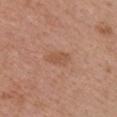<lesion>
<biopsy_status>not biopsied; imaged during a skin examination</biopsy_status>
<image>
  <source>total-body photography crop</source>
  <field_of_view_mm>15</field_of_view_mm>
</image>
<lighting>white-light</lighting>
<site>chest</site>
<patient>
  <sex>female</sex>
  <age_approx>50</age_approx>
</patient>
<automated_metrics>
  <area_mm2_approx>4.5</area_mm2_approx>
  <eccentricity>0.85</eccentricity>
  <cielab_L>53</cielab_L>
  <cielab_a>22</cielab_a>
  <cielab_b>32</cielab_b>
  <vs_skin_darker_L>7.0</vs_skin_darker_L>
  <vs_skin_contrast_norm>5.5</vs_skin_contrast_norm>
  <border_irregularity_0_10>2.5</border_irregularity_0_10>
</automated_metrics>
</lesion>